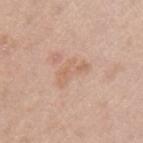Recorded during total-body skin imaging; not selected for excision or biopsy.
A region of skin cropped from a whole-body photographic capture, roughly 15 mm wide.
The total-body-photography lesion software estimated a footprint of about 7 mm², a shape eccentricity near 0.85, and a shape-asymmetry score of about 0.45 (0 = symmetric). It also reported border irregularity of about 4.5 on a 0–10 scale, a within-lesion color-variation index near 2.5/10, and peripheral color asymmetry of about 1.
This is a white-light tile.
A female patient aged approximately 50.
Longest diameter approximately 4 mm.
From the left upper arm.